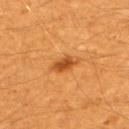No biopsy was performed on this lesion — it was imaged during a full skin examination and was not determined to be concerning. A male subject aged 58 to 62. The lesion's longest dimension is about 3.5 mm. Automated image analysis of the tile measured a lesion color around L≈48 a*≈27 b*≈44 in CIELAB, a lesion–skin lightness drop of about 11, and a lesion-to-skin contrast of about 8 (normalized; higher = more distinct). The software also gave border irregularity of about 2.5 on a 0–10 scale, internal color variation of about 2.5 on a 0–10 scale, and peripheral color asymmetry of about 0.5. The lesion is located on the upper back. A close-up tile cropped from a whole-body skin photograph, about 15 mm across. This is a cross-polarized tile.